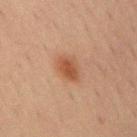| key | value |
|---|---|
| follow-up | catalogued during a skin exam; not biopsied |
| subject | male, aged 68–72 |
| lighting | cross-polarized |
| imaging modality | 15 mm crop, total-body photography |
| site | the front of the torso |
| size | ~3.5 mm (longest diameter) |
| automated lesion analysis | a nevus-likeness score of about 95/100 and lesion-presence confidence of about 100/100 |